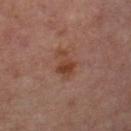Captured during whole-body skin photography for melanoma surveillance; the lesion was not biopsied.